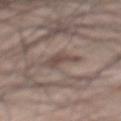<case>
  <biopsy_status>not biopsied; imaged during a skin examination</biopsy_status>
  <lesion_size>
    <long_diameter_mm_approx>3.0</long_diameter_mm_approx>
  </lesion_size>
  <automated_metrics>
    <area_mm2_approx>5.5</area_mm2_approx>
    <eccentricity>0.7</eccentricity>
    <shape_asymmetry>0.5</shape_asymmetry>
    <cielab_L>47</cielab_L>
    <cielab_a>14</cielab_a>
    <cielab_b>20</cielab_b>
    <vs_skin_darker_L>9.0</vs_skin_darker_L>
    <nevus_likeness_0_100>0</nevus_likeness_0_100>
    <lesion_detection_confidence_0_100>95</lesion_detection_confidence_0_100>
  </automated_metrics>
  <image>
    <source>total-body photography crop</source>
    <field_of_view_mm>15</field_of_view_mm>
  </image>
  <site>back</site>
  <patient>
    <sex>male</sex>
    <age_approx>70</age_approx>
  </patient>
</case>